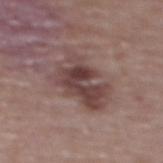<lesion>
<biopsy_status>not biopsied; imaged during a skin examination</biopsy_status>
<image>
  <source>total-body photography crop</source>
  <field_of_view_mm>15</field_of_view_mm>
</image>
<patient>
  <sex>female</sex>
  <age_approx>65</age_approx>
</patient>
<lighting>white-light</lighting>
<site>upper back</site>
</lesion>